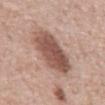Assessment:
No biopsy was performed on this lesion — it was imaged during a full skin examination and was not determined to be concerning.
Image and clinical context:
A close-up tile cropped from a whole-body skin photograph, about 15 mm across. The lesion is on the abdomen. The subject is a male aged 58–62. An algorithmic analysis of the crop reported a lesion color around L≈53 a*≈20 b*≈25 in CIELAB and about 14 CIELAB-L* units darker than the surrounding skin. The software also gave a border-irregularity index near 1.5/10, a within-lesion color-variation index near 5/10, and peripheral color asymmetry of about 2. The analysis additionally found a classifier nevus-likeness of about 95/100 and a detector confidence of about 100 out of 100 that the crop contains a lesion. The recorded lesion diameter is about 6.5 mm. Captured under white-light illumination.